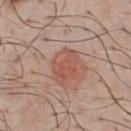The lesion was photographed on a routine skin check and not biopsied; there is no pathology result. About 4 mm across. A close-up tile cropped from a whole-body skin photograph, about 15 mm across. This is a white-light tile. Automated image analysis of the tile measured a footprint of about 9.5 mm², a shape eccentricity near 0.7, and a shape-asymmetry score of about 0.2 (0 = symmetric). It also reported a mean CIELAB color near L≈54 a*≈24 b*≈28, a lesion–skin lightness drop of about 8, and a normalized border contrast of about 6. The analysis additionally found a classifier nevus-likeness of about 90/100 and a lesion-detection confidence of about 100/100. A male patient about 45 years old. On the chest.